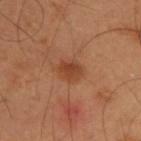Q: Was this lesion biopsied?
A: no biopsy performed (imaged during a skin exam)
Q: What is the anatomic site?
A: the upper back
Q: Patient demographics?
A: male, in their mid-50s
Q: What kind of image is this?
A: 15 mm crop, total-body photography